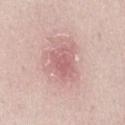Impression:
This lesion was catalogued during total-body skin photography and was not selected for biopsy.
Acquisition and patient details:
A female subject roughly 40 years of age. The lesion's longest dimension is about 4.5 mm. A 15 mm close-up tile from a total-body photography series done for melanoma screening. This is a white-light tile. The lesion is on the abdomen.